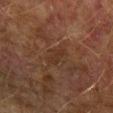Captured during whole-body skin photography for melanoma surveillance; the lesion was not biopsied.
This is a cross-polarized tile.
The subject is a male about 75 years old.
A close-up tile cropped from a whole-body skin photograph, about 15 mm across.
From the left forearm.
The lesion's longest dimension is about 2.5 mm.
The lesion-visualizer software estimated an area of roughly 2.5 mm², a shape eccentricity near 0.9, and two-axis asymmetry of about 0.3. The analysis additionally found a detector confidence of about 90 out of 100 that the crop contains a lesion.